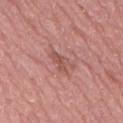Impression: Captured during whole-body skin photography for melanoma surveillance; the lesion was not biopsied. Image and clinical context: The recorded lesion diameter is about 4 mm. The subject is a male in their 80s. On the lower back. A lesion tile, about 15 mm wide, cut from a 3D total-body photograph.